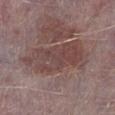From the left lower leg.
Cropped from a total-body skin-imaging series; the visible field is about 15 mm.
A male subject about 75 years old.